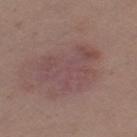subject = female, about 30 years old; lighting = white-light; imaging modality = 15 mm crop, total-body photography; anatomic site = the left thigh; histopathology = an invasive melanoma arising in association with a nevus, Breslow thickness 0.4 mm — a malignant lesion.